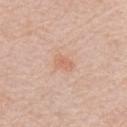Part of a total-body skin-imaging series; this lesion was reviewed on a skin check and was not flagged for biopsy. The patient is a female aged around 65. Cropped from a whole-body photographic skin survey; the tile spans about 15 mm. The lesion is located on the arm. The total-body-photography lesion software estimated border irregularity of about 2 on a 0–10 scale and a color-variation rating of about 1.5/10. The analysis additionally found a nevus-likeness score of about 10/100 and a detector confidence of about 100 out of 100 that the crop contains a lesion.